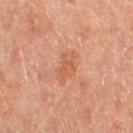{
  "biopsy_status": "not biopsied; imaged during a skin examination",
  "image": {
    "source": "total-body photography crop",
    "field_of_view_mm": 15
  },
  "site": "leg",
  "lesion_size": {
    "long_diameter_mm_approx": 4.0
  },
  "lighting": "cross-polarized",
  "patient": {
    "sex": "female",
    "age_approx": 55
  }
}